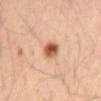Approximately 2.5 mm at its widest. Automated image analysis of the tile measured a border-irregularity rating of about 1.5/10, internal color variation of about 7.5 on a 0–10 scale, and a peripheral color-asymmetry measure near 2.5. The software also gave a nevus-likeness score of about 100/100 and lesion-presence confidence of about 100/100. The tile uses cross-polarized illumination. The lesion is located on the mid back. A region of skin cropped from a whole-body photographic capture, roughly 15 mm wide. A male subject, aged approximately 50.